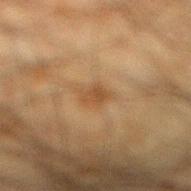Notes:
* lighting: cross-polarized
* size: ~2.5 mm (longest diameter)
* image source: 15 mm crop, total-body photography
* patient: male, in their mid-30s
* automated metrics: a peripheral color-asymmetry measure near 1; a classifier nevus-likeness of about 30/100 and lesion-presence confidence of about 100/100
* body site: the right lower leg